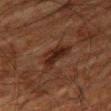Case summary:
- follow-up · total-body-photography surveillance lesion; no biopsy
- location · the right thigh
- patient · male, aged 78–82
- illumination · cross-polarized illumination
- image source · ~15 mm crop, total-body skin-cancer survey
- diameter · ~4.5 mm (longest diameter)
- automated metrics · an area of roughly 8 mm² and a shape-asymmetry score of about 0.4 (0 = symmetric); a color-variation rating of about 3.5/10 and radial color variation of about 1